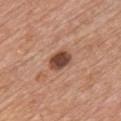body site: the chest
lesion size: ≈3 mm
lighting: white-light
patient: male, about 60 years old
image source: 15 mm crop, total-body photography